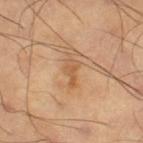{"biopsy_status": "not biopsied; imaged during a skin examination", "lesion_size": {"long_diameter_mm_approx": 3.0}, "image": {"source": "total-body photography crop", "field_of_view_mm": 15}, "lighting": "cross-polarized", "patient": {"sex": "male", "age_approx": 60}, "site": "right thigh"}